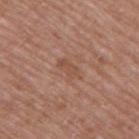The lesion was tiled from a total-body skin photograph and was not biopsied.
The lesion-visualizer software estimated a classifier nevus-likeness of about 0/100 and a detector confidence of about 100 out of 100 that the crop contains a lesion.
A close-up tile cropped from a whole-body skin photograph, about 15 mm across.
The lesion's longest dimension is about 3.5 mm.
The lesion is on the upper back.
This is a white-light tile.
The subject is a female about 60 years old.